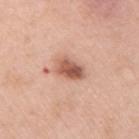Clinical impression: The lesion was tiled from a total-body skin photograph and was not biopsied. Image and clinical context: Imaged with white-light lighting. Automated tile analysis of the lesion measured a shape eccentricity near 0.75 and two-axis asymmetry of about 0.15. The analysis additionally found a border-irregularity index near 2/10 and peripheral color asymmetry of about 2.5. The software also gave a nevus-likeness score of about 95/100 and lesion-presence confidence of about 100/100. On the right upper arm. About 3.5 mm across. The subject is a male in their 50s. A 15 mm crop from a total-body photograph taken for skin-cancer surveillance.